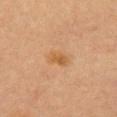Q: Is there a histopathology result?
A: no biopsy performed (imaged during a skin exam)
Q: How was the tile lit?
A: cross-polarized
Q: How was this image acquired?
A: total-body-photography crop, ~15 mm field of view
Q: What is the anatomic site?
A: the chest
Q: Who is the patient?
A: female, roughly 65 years of age
Q: What is the lesion's diameter?
A: ~2.5 mm (longest diameter)